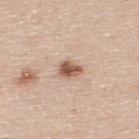biopsy_status: not biopsied; imaged during a skin examination
image:
  source: total-body photography crop
  field_of_view_mm: 15
lesion_size:
  long_diameter_mm_approx: 2.5
automated_metrics:
  cielab_L: 56
  cielab_a: 20
  cielab_b: 29
  vs_skin_darker_L: 15.0
  vs_skin_contrast_norm: 10.0
  border_irregularity_0_10: 3.0
  color_variation_0_10: 3.5
  nevus_likeness_0_100: 95
  lesion_detection_confidence_0_100: 100
patient:
  sex: male
  age_approx: 45
lighting: white-light
site: upper back